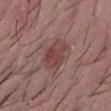{"biopsy_status": "not biopsied; imaged during a skin examination", "lighting": "white-light", "patient": {"sex": "male", "age_approx": 30}, "lesion_size": {"long_diameter_mm_approx": 4.5}, "image": {"source": "total-body photography crop", "field_of_view_mm": 15}}